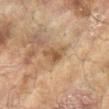Notes:
- notes: total-body-photography surveillance lesion; no biopsy
- size: about 3 mm
- acquisition: total-body-photography crop, ~15 mm field of view
- site: the right arm
- patient: female, aged approximately 80
- illumination: cross-polarized illumination